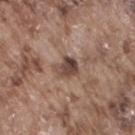workup=total-body-photography surveillance lesion; no biopsy
patient=male, about 75 years old
site=the right thigh
diameter=about 3 mm
imaging modality=15 mm crop, total-body photography
lighting=white-light